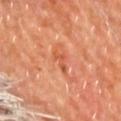The lesion was photographed on a routine skin check and not biopsied; there is no pathology result. A male patient, aged 58 to 62. Imaged with cross-polarized lighting. Approximately 2.5 mm at its widest. The lesion is on the upper back. Cropped from a total-body skin-imaging series; the visible field is about 15 mm. The total-body-photography lesion software estimated border irregularity of about 6.5 on a 0–10 scale, internal color variation of about 0 on a 0–10 scale, and a peripheral color-asymmetry measure near 0.